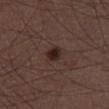Part of a total-body skin-imaging series; this lesion was reviewed on a skin check and was not flagged for biopsy. The lesion is on the right thigh. Captured under white-light illumination. A male patient about 50 years old. Automated image analysis of the tile measured a mean CIELAB color near L≈24 a*≈15 b*≈18 and a normalized border contrast of about 10. It also reported an automated nevus-likeness rating near 90 out of 100 and a detector confidence of about 100 out of 100 that the crop contains a lesion. The recorded lesion diameter is about 2.5 mm. A 15 mm close-up extracted from a 3D total-body photography capture.